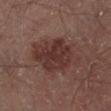Notes:
- workup — catalogued during a skin exam; not biopsied
- subject — male, aged 48–52
- illumination — cross-polarized illumination
- anatomic site — the right lower leg
- size — about 7 mm
- image — ~15 mm crop, total-body skin-cancer survey